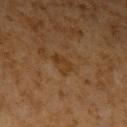Findings:
– biopsy status · catalogued during a skin exam; not biopsied
– body site · the arm
– illumination · cross-polarized
– subject · male, roughly 60 years of age
– imaging modality · total-body-photography crop, ~15 mm field of view
– lesion size · about 2.5 mm